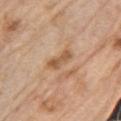Clinical impression:
This lesion was catalogued during total-body skin photography and was not selected for biopsy.
Acquisition and patient details:
Automated image analysis of the tile measured an area of roughly 5 mm² and a shape-asymmetry score of about 0.3 (0 = symmetric). The analysis additionally found roughly 10 lightness units darker than nearby skin and a normalized border contrast of about 7.5. The analysis additionally found internal color variation of about 2.5 on a 0–10 scale and peripheral color asymmetry of about 0.5. It also reported an automated nevus-likeness rating near 0 out of 100 and a detector confidence of about 100 out of 100 that the crop contains a lesion. About 4 mm across. On the left upper arm. A close-up tile cropped from a whole-body skin photograph, about 15 mm across. Captured under white-light illumination. The subject is a male roughly 70 years of age.